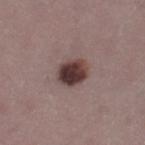Impression:
Recorded during total-body skin imaging; not selected for excision or biopsy.
Background:
This image is a 15 mm lesion crop taken from a total-body photograph. The patient is a female roughly 45 years of age. Approximately 3.5 mm at its widest. The lesion-visualizer software estimated an area of roughly 8.5 mm², a shape eccentricity near 0.6, and two-axis asymmetry of about 0.15. It also reported a lesion-detection confidence of about 100/100. This is a white-light tile. Located on the left thigh.